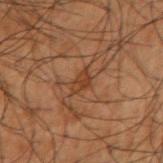biopsy status=total-body-photography surveillance lesion; no biopsy
patient=male, in their 50s
lesion size=≈2.5 mm
lighting=cross-polarized
body site=the right upper arm
acquisition=total-body-photography crop, ~15 mm field of view